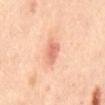No biopsy was performed on this lesion — it was imaged during a full skin examination and was not determined to be concerning.